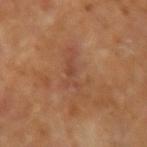Clinical impression: The lesion was photographed on a routine skin check and not biopsied; there is no pathology result. Context: The subject is a female aged around 60. This is a cross-polarized tile. The recorded lesion diameter is about 5.5 mm. From the right forearm. The lesion-visualizer software estimated an average lesion color of about L≈46 a*≈22 b*≈31 (CIELAB) and a lesion-to-skin contrast of about 4.5 (normalized; higher = more distinct). Cropped from a total-body skin-imaging series; the visible field is about 15 mm.